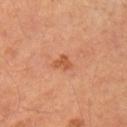Q: Was a biopsy performed?
A: catalogued during a skin exam; not biopsied
Q: What lighting was used for the tile?
A: cross-polarized
Q: What is the anatomic site?
A: the right upper arm
Q: What is the imaging modality?
A: ~15 mm crop, total-body skin-cancer survey
Q: Who is the patient?
A: male, aged around 65